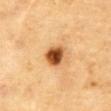{
  "biopsy_status": "not biopsied; imaged during a skin examination",
  "patient": {
    "sex": "male",
    "age_approx": "85+"
  },
  "lighting": "cross-polarized",
  "site": "abdomen",
  "image": {
    "source": "total-body photography crop",
    "field_of_view_mm": 15
  }
}